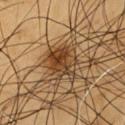Findings:
• image source: ~15 mm crop, total-body skin-cancer survey
• patient: male, approximately 60 years of age
• automated lesion analysis: an area of roughly 12 mm², an outline eccentricity of about 0.45 (0 = round, 1 = elongated), and a shape-asymmetry score of about 0.25 (0 = symmetric); a lesion color around L≈42 a*≈18 b*≈35 in CIELAB
• lesion diameter: about 4.5 mm
• body site: the chest
• tile lighting: cross-polarized